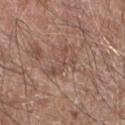• workup · imaged on a skin check; not biopsied